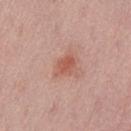<record>
<biopsy_status>not biopsied; imaged during a skin examination</biopsy_status>
<image>
  <source>total-body photography crop</source>
  <field_of_view_mm>15</field_of_view_mm>
</image>
<site>left thigh</site>
<patient>
  <sex>male</sex>
  <age_approx>40</age_approx>
</patient>
</record>